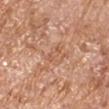Acquisition and patient details:
The subject is a male in their mid- to late 60s. A region of skin cropped from a whole-body photographic capture, roughly 15 mm wide. About 2.5 mm across. The lesion is located on the right upper arm.